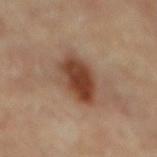Clinical impression: The lesion was photographed on a routine skin check and not biopsied; there is no pathology result. Background: The subject is a male roughly 85 years of age or older. This is a cross-polarized tile. A 15 mm close-up extracted from a 3D total-body photography capture. Located on the mid back.